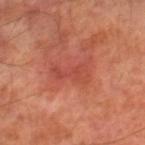Q: Is there a histopathology result?
A: total-body-photography surveillance lesion; no biopsy
Q: Patient demographics?
A: male, about 70 years old
Q: Lesion size?
A: ~4.5 mm (longest diameter)
Q: Lesion location?
A: the right lower leg
Q: What is the imaging modality?
A: ~15 mm tile from a whole-body skin photo